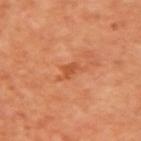Q: Was a biopsy performed?
A: imaged on a skin check; not biopsied
Q: Patient demographics?
A: female, aged around 50
Q: What kind of image is this?
A: 15 mm crop, total-body photography
Q: Lesion location?
A: the left upper arm